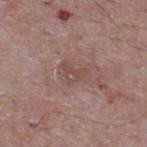Q: Where on the body is the lesion?
A: the back
Q: Lesion size?
A: ≈3 mm
Q: How was the tile lit?
A: white-light illumination
Q: Patient demographics?
A: male, in their mid-60s
Q: What kind of image is this?
A: 15 mm crop, total-body photography